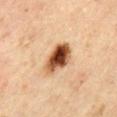Background:
A male patient aged approximately 45. Automated image analysis of the tile measured a lesion color around L≈44 a*≈21 b*≈32 in CIELAB and a normalized lesion–skin contrast near 14. The software also gave an automated nevus-likeness rating near 100 out of 100. This image is a 15 mm lesion crop taken from a total-body photograph. About 4.5 mm across. Located on the mid back. This is a cross-polarized tile.